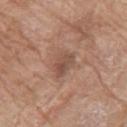* diameter: about 3.5 mm
* body site: the leg
* imaging modality: ~15 mm tile from a whole-body skin photo
* automated lesion analysis: a lesion area of about 6.5 mm², an outline eccentricity of about 0.75 (0 = round, 1 = elongated), and two-axis asymmetry of about 0.3; a nevus-likeness score of about 0/100 and a lesion-detection confidence of about 100/100
* patient: female, in their mid-70s